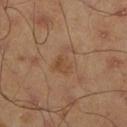<case>
  <site>right lower leg</site>
  <image>
    <source>total-body photography crop</source>
    <field_of_view_mm>15</field_of_view_mm>
  </image>
  <patient>
    <sex>male</sex>
    <age_approx>45</age_approx>
  </patient>
</case>